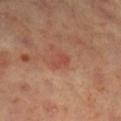Acquisition and patient details:
The patient is a female aged 68 to 72. About 2.5 mm across. This is a cross-polarized tile. Located on the leg. Cropped from a whole-body photographic skin survey; the tile spans about 15 mm.